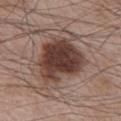Background:
From the chest. About 6 mm across. The tile uses white-light illumination. A close-up tile cropped from a whole-body skin photograph, about 15 mm across. A male subject, roughly 65 years of age. The total-body-photography lesion software estimated an average lesion color of about L≈39 a*≈18 b*≈23 (CIELAB). The software also gave border irregularity of about 3 on a 0–10 scale, a color-variation rating of about 5/10, and a peripheral color-asymmetry measure near 1.5. The software also gave an automated nevus-likeness rating near 80 out of 100 and lesion-presence confidence of about 100/100.